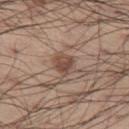biopsy status=catalogued during a skin exam; not biopsied
acquisition=~15 mm tile from a whole-body skin photo
lesion size=≈3 mm
location=the left thigh
subject=male, aged approximately 55